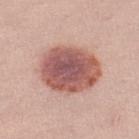Clinical impression: Imaged during a routine full-body skin examination; the lesion was not biopsied and no histopathology is available. Background: A female patient in their mid- to late 20s. Imaged with white-light lighting. A roughly 15 mm field-of-view crop from a total-body skin photograph. The lesion is on the leg. Longest diameter approximately 7 mm. The total-body-photography lesion software estimated a footprint of about 29 mm², an eccentricity of roughly 0.6, and two-axis asymmetry of about 0.1.